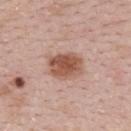The lesion was photographed on a routine skin check and not biopsied; there is no pathology result. A close-up tile cropped from a whole-body skin photograph, about 15 mm across. A female patient, aged 28–32. The lesion is on the upper back. About 4.5 mm across. Automated image analysis of the tile measured an average lesion color of about L≈54 a*≈22 b*≈29 (CIELAB), a lesion–skin lightness drop of about 13, and a normalized border contrast of about 9.5. The software also gave border irregularity of about 2 on a 0–10 scale, a color-variation rating of about 4.5/10, and peripheral color asymmetry of about 1.5. Imaged with white-light lighting.